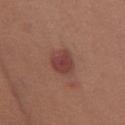Q: Is there a histopathology result?
A: no biopsy performed (imaged during a skin exam)